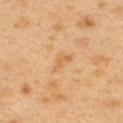On the upper back. An algorithmic analysis of the crop reported a mean CIELAB color near L≈51 a*≈17 b*≈36 and a lesion-to-skin contrast of about 5.5 (normalized; higher = more distinct). And it measured a classifier nevus-likeness of about 0/100 and a lesion-detection confidence of about 100/100. A 15 mm crop from a total-body photograph taken for skin-cancer surveillance. A female patient, about 40 years old. Captured under cross-polarized illumination.